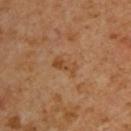Assessment:
The lesion was tiled from a total-body skin photograph and was not biopsied.
Background:
A male patient aged approximately 60. Cropped from a total-body skin-imaging series; the visible field is about 15 mm. This is a cross-polarized tile. The lesion is on the upper back.